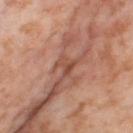This lesion was catalogued during total-body skin photography and was not selected for biopsy.